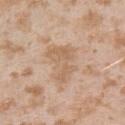No biopsy was performed on this lesion — it was imaged during a full skin examination and was not determined to be concerning.
The recorded lesion diameter is about 5 mm.
A female subject in their mid- to late 20s.
This is a white-light tile.
From the left upper arm.
A lesion tile, about 15 mm wide, cut from a 3D total-body photograph.